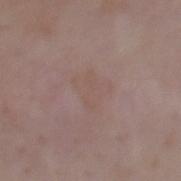notes = imaged on a skin check; not biopsied
patient = female, roughly 45 years of age
image source = 15 mm crop, total-body photography
site = the mid back